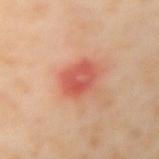workup — catalogued during a skin exam; not biopsied | subject — female, aged around 55 | body site — the arm | TBP lesion metrics — a mean CIELAB color near L≈58 a*≈35 b*≈33, roughly 11 lightness units darker than nearby skin, and a normalized lesion–skin contrast near 7; a detector confidence of about 100 out of 100 that the crop contains a lesion | lesion size — about 3.5 mm | image source — total-body-photography crop, ~15 mm field of view.